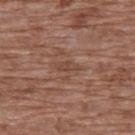The lesion was photographed on a routine skin check and not biopsied; there is no pathology result. A female patient, about 75 years old. Captured under white-light illumination. From the back. A lesion tile, about 15 mm wide, cut from a 3D total-body photograph. Approximately 3 mm at its widest.